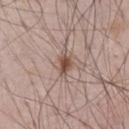Assessment:
This lesion was catalogued during total-body skin photography and was not selected for biopsy.
Context:
Cropped from a total-body skin-imaging series; the visible field is about 15 mm. The lesion is on the front of the torso. This is a white-light tile. The subject is a male in their mid-60s. Measured at roughly 3 mm in maximum diameter.